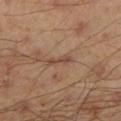Imaged during a routine full-body skin examination; the lesion was not biopsied and no histopathology is available. A male patient roughly 45 years of age. Captured under cross-polarized illumination. Measured at roughly 3 mm in maximum diameter. On the left lower leg. A region of skin cropped from a whole-body photographic capture, roughly 15 mm wide. Automated tile analysis of the lesion measured a lesion area of about 3 mm², an eccentricity of roughly 0.95, and a shape-asymmetry score of about 0.4 (0 = symmetric). It also reported a mean CIELAB color near L≈45 a*≈19 b*≈27, about 8 CIELAB-L* units darker than the surrounding skin, and a normalized lesion–skin contrast near 6.5. The software also gave a color-variation rating of about 0/10 and radial color variation of about 0.